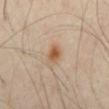Q: Is there a histopathology result?
A: no biopsy performed (imaged during a skin exam)
Q: Lesion size?
A: about 2.5 mm
Q: What kind of image is this?
A: ~15 mm tile from a whole-body skin photo
Q: Patient demographics?
A: male, approximately 40 years of age
Q: How was the tile lit?
A: cross-polarized
Q: Automated lesion metrics?
A: border irregularity of about 2.5 on a 0–10 scale, internal color variation of about 3 on a 0–10 scale, and a peripheral color-asymmetry measure near 0.5; a nevus-likeness score of about 95/100 and a detector confidence of about 100 out of 100 that the crop contains a lesion
Q: What is the anatomic site?
A: the mid back